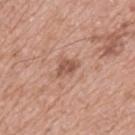Q: Is there a histopathology result?
A: catalogued during a skin exam; not biopsied
Q: Automated lesion metrics?
A: a footprint of about 4 mm² and a symmetry-axis asymmetry near 0.4; a lesion color around L≈54 a*≈22 b*≈29 in CIELAB, roughly 10 lightness units darker than nearby skin, and a normalized lesion–skin contrast near 7; a nevus-likeness score of about 25/100
Q: How large is the lesion?
A: about 3 mm
Q: Lesion location?
A: the upper back
Q: Patient demographics?
A: male, aged around 75
Q: What kind of image is this?
A: 15 mm crop, total-body photography